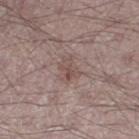Q: Was a biopsy performed?
A: total-body-photography surveillance lesion; no biopsy
Q: How was the tile lit?
A: white-light
Q: What kind of image is this?
A: ~15 mm tile from a whole-body skin photo
Q: Who is the patient?
A: male, aged around 50
Q: How large is the lesion?
A: ~3 mm (longest diameter)
Q: Where on the body is the lesion?
A: the right thigh